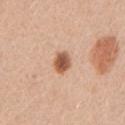The lesion was tiled from a total-body skin photograph and was not biopsied.
A female patient, aged around 45.
A 15 mm crop from a total-body photograph taken for skin-cancer surveillance.
Located on the left upper arm.